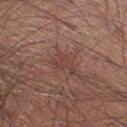The lesion was photographed on a routine skin check and not biopsied; there is no pathology result. Longest diameter approximately 3.5 mm. The lesion is located on the right thigh. The subject is a male about 45 years old. A 15 mm close-up tile from a total-body photography series done for melanoma screening.